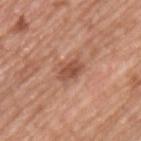The lesion was tiled from a total-body skin photograph and was not biopsied. A lesion tile, about 15 mm wide, cut from a 3D total-body photograph. Automated image analysis of the tile measured a lesion–skin lightness drop of about 11 and a lesion-to-skin contrast of about 7.5 (normalized; higher = more distinct). And it measured border irregularity of about 3 on a 0–10 scale and a within-lesion color-variation index near 2.5/10. Imaged with white-light lighting. A male subject, aged approximately 50. Measured at roughly 3 mm in maximum diameter. The lesion is located on the upper back.